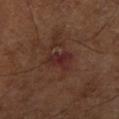workup = imaged on a skin check; not biopsied
location = the right lower leg
imaging modality = ~15 mm tile from a whole-body skin photo
patient = approximately 65 years of age
lighting = cross-polarized
lesion diameter = ≈3.5 mm